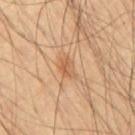The lesion was photographed on a routine skin check and not biopsied; there is no pathology result. A male subject aged around 55. A close-up tile cropped from a whole-body skin photograph, about 15 mm across. The recorded lesion diameter is about 2.5 mm. Automated tile analysis of the lesion measured an average lesion color of about L≈57 a*≈21 b*≈36 (CIELAB), about 8 CIELAB-L* units darker than the surrounding skin, and a lesion-to-skin contrast of about 6 (normalized; higher = more distinct). And it measured a border-irregularity rating of about 3/10, a within-lesion color-variation index near 3/10, and radial color variation of about 1. The software also gave a nevus-likeness score of about 0/100 and lesion-presence confidence of about 100/100. Located on the mid back.